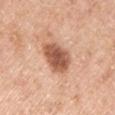workup = catalogued during a skin exam; not biopsied | imaging modality = total-body-photography crop, ~15 mm field of view | anatomic site = the left upper arm | patient = male, in their mid- to late 60s.